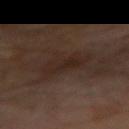* workup — no biopsy performed (imaged during a skin exam)
* patient — male, aged 68 to 72
* location — the arm
* imaging modality — ~15 mm tile from a whole-body skin photo
* diameter — ≈4.5 mm